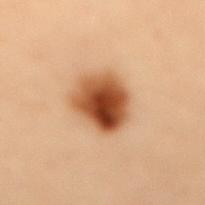No biopsy was performed on this lesion — it was imaged during a full skin examination and was not determined to be concerning.
The patient is a female approximately 50 years of age.
From the mid back.
A lesion tile, about 15 mm wide, cut from a 3D total-body photograph.